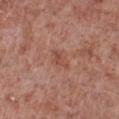This lesion was catalogued during total-body skin photography and was not selected for biopsy. The tile uses white-light illumination. Measured at roughly 3 mm in maximum diameter. A male subject in their mid-50s. The lesion-visualizer software estimated an outline eccentricity of about 0.85 (0 = round, 1 = elongated) and a symmetry-axis asymmetry near 0.4. It also reported an average lesion color of about L≈49 a*≈24 b*≈28 (CIELAB), about 7 CIELAB-L* units darker than the surrounding skin, and a normalized lesion–skin contrast near 5.5. And it measured border irregularity of about 4.5 on a 0–10 scale and peripheral color asymmetry of about 1. Cropped from a whole-body photographic skin survey; the tile spans about 15 mm. The lesion is located on the left lower leg.